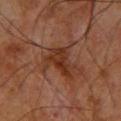This lesion was catalogued during total-body skin photography and was not selected for biopsy.
On the upper back.
This image is a 15 mm lesion crop taken from a total-body photograph.
Longest diameter approximately 4 mm.
A male patient, aged approximately 60.
Imaged with cross-polarized lighting.